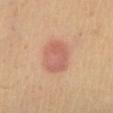Findings:
• workup — catalogued during a skin exam; not biopsied
• TBP lesion metrics — an area of roughly 13 mm², an eccentricity of roughly 0.7, and a symmetry-axis asymmetry near 0.1; a mean CIELAB color near L≈61 a*≈25 b*≈30, a lesion–skin lightness drop of about 9, and a normalized lesion–skin contrast near 6.5; a border-irregularity rating of about 1.5/10, a color-variation rating of about 3.5/10, and peripheral color asymmetry of about 1
• lesion diameter — ≈4.5 mm
• illumination — cross-polarized
• imaging modality — total-body-photography crop, ~15 mm field of view
• subject — female, roughly 40 years of age
• location — the left lower leg